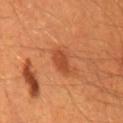This lesion was catalogued during total-body skin photography and was not selected for biopsy. The recorded lesion diameter is about 3 mm. The lesion is located on the mid back. A roughly 15 mm field-of-view crop from a total-body skin photograph. Imaged with cross-polarized lighting. A male patient, aged approximately 60.